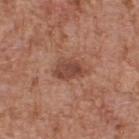The lesion was tiled from a total-body skin photograph and was not biopsied. This image is a 15 mm lesion crop taken from a total-body photograph. A male subject roughly 65 years of age. An algorithmic analysis of the crop reported a mean CIELAB color near L≈46 a*≈23 b*≈27, a lesion–skin lightness drop of about 10, and a normalized lesion–skin contrast near 7.5. The software also gave a border-irregularity rating of about 3/10 and peripheral color asymmetry of about 1. It also reported a nevus-likeness score of about 60/100 and a detector confidence of about 100 out of 100 that the crop contains a lesion. Imaged with white-light lighting. From the back. Approximately 4 mm at its widest.